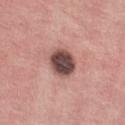Q: Was this lesion biopsied?
A: no biopsy performed (imaged during a skin exam)
Q: How large is the lesion?
A: about 3.5 mm
Q: Where on the body is the lesion?
A: the left forearm
Q: What lighting was used for the tile?
A: white-light illumination
Q: What is the imaging modality?
A: total-body-photography crop, ~15 mm field of view
Q: Patient demographics?
A: male, roughly 25 years of age